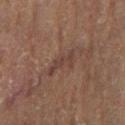Assessment: Captured during whole-body skin photography for melanoma surveillance; the lesion was not biopsied. Context: A male subject aged 53–57. Located on the right lower leg. This is a cross-polarized tile. This image is a 15 mm lesion crop taken from a total-body photograph. An algorithmic analysis of the crop reported a border-irregularity rating of about 7/10 and radial color variation of about 0.5.